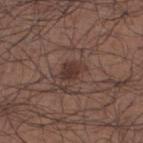Impression:
Part of a total-body skin-imaging series; this lesion was reviewed on a skin check and was not flagged for biopsy.
Clinical summary:
This is a white-light tile. On the leg. The recorded lesion diameter is about 3 mm. A 15 mm crop from a total-body photograph taken for skin-cancer surveillance. A male patient roughly 65 years of age. The total-body-photography lesion software estimated a lesion area of about 4.5 mm², an outline eccentricity of about 0.7 (0 = round, 1 = elongated), and a shape-asymmetry score of about 0.3 (0 = symmetric). The analysis additionally found border irregularity of about 3 on a 0–10 scale, a color-variation rating of about 1.5/10, and radial color variation of about 0.5. It also reported a nevus-likeness score of about 70/100.